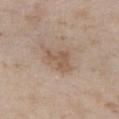notes — imaged on a skin check; not biopsied
body site — the front of the torso
TBP lesion metrics — a border-irregularity index near 5.5/10, a within-lesion color-variation index near 1.5/10, and a peripheral color-asymmetry measure near 0.5
acquisition — total-body-photography crop, ~15 mm field of view
patient — female, approximately 30 years of age